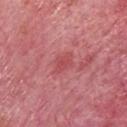Q: Was this lesion biopsied?
A: total-body-photography surveillance lesion; no biopsy
Q: What is the imaging modality?
A: ~15 mm crop, total-body skin-cancer survey
Q: What are the patient's age and sex?
A: female, aged around 60
Q: How was the tile lit?
A: white-light
Q: Where on the body is the lesion?
A: the head or neck
Q: How large is the lesion?
A: ~3 mm (longest diameter)